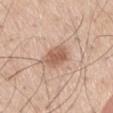This image is a 15 mm lesion crop taken from a total-body photograph.
The patient is a male aged around 60.
From the right thigh.